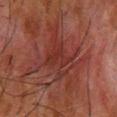follow-up = catalogued during a skin exam; not biopsied | subject = male, in their mid- to late 60s | body site = the upper back | image = 15 mm crop, total-body photography.